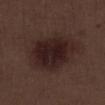* notes · catalogued during a skin exam; not biopsied
* acquisition · total-body-photography crop, ~15 mm field of view
* size · about 6.5 mm
* site · the leg
* illumination · white-light illumination
* subject · male, aged 68 to 72